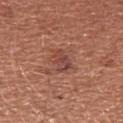{
  "biopsy_status": "not biopsied; imaged during a skin examination",
  "site": "arm",
  "patient": {
    "sex": "female",
    "age_approx": 35
  },
  "lesion_size": {
    "long_diameter_mm_approx": 3.0
  },
  "image": {
    "source": "total-body photography crop",
    "field_of_view_mm": 15
  }
}